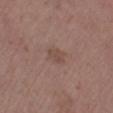Findings:
- notes — total-body-photography surveillance lesion; no biopsy
- site — the right lower leg
- imaging modality — ~15 mm crop, total-body skin-cancer survey
- size — ≈3 mm
- patient — male, about 70 years old